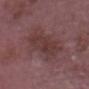Recorded during total-body skin imaging; not selected for excision or biopsy. Approximately 8 mm at its widest. The lesion is located on the head or neck. A male patient, in their mid-60s. Imaged with white-light lighting. A 15 mm close-up tile from a total-body photography series done for melanoma screening.